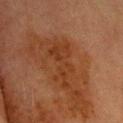Case summary:
• biopsy status: catalogued during a skin exam; not biopsied
• location: the head or neck
• tile lighting: cross-polarized
• patient: male, aged approximately 70
• imaging modality: 15 mm crop, total-body photography
• size: ~7 mm (longest diameter)